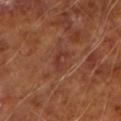Imaged during a routine full-body skin examination; the lesion was not biopsied and no histopathology is available.
A male subject in their mid-60s.
The total-body-photography lesion software estimated a footprint of about 3 mm², an eccentricity of roughly 0.85, and two-axis asymmetry of about 0.55. It also reported an average lesion color of about L≈34 a*≈25 b*≈25 (CIELAB), roughly 6 lightness units darker than nearby skin, and a lesion-to-skin contrast of about 6 (normalized; higher = more distinct). The analysis additionally found a border-irregularity index near 6.5/10, a color-variation rating of about 0/10, and a peripheral color-asymmetry measure near 0.
A 15 mm close-up extracted from a 3D total-body photography capture.
On the left upper arm.
Captured under cross-polarized illumination.